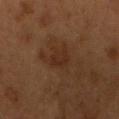Impression: Recorded during total-body skin imaging; not selected for excision or biopsy. Clinical summary: Cropped from a total-body skin-imaging series; the visible field is about 15 mm. Measured at roughly 2.5 mm in maximum diameter. Captured under cross-polarized illumination. The patient is a male aged 53 to 57. The lesion is located on the head or neck.